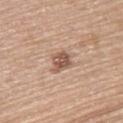Assessment:
Part of a total-body skin-imaging series; this lesion was reviewed on a skin check and was not flagged for biopsy.
Image and clinical context:
An algorithmic analysis of the crop reported a footprint of about 5.5 mm² and a shape-asymmetry score of about 0.3 (0 = symmetric). It also reported a border-irregularity index near 3/10. The analysis additionally found lesion-presence confidence of about 100/100. A 15 mm close-up tile from a total-body photography series done for melanoma screening. The lesion is located on the upper back. The patient is a female roughly 65 years of age. Imaged with white-light lighting. About 3 mm across.